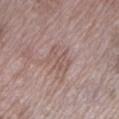The lesion was photographed on a routine skin check and not biopsied; there is no pathology result. Located on the leg. Cropped from a whole-body photographic skin survey; the tile spans about 15 mm. The patient is a female aged 68–72. The total-body-photography lesion software estimated an outline eccentricity of about 0.7 (0 = round, 1 = elongated) and a shape-asymmetry score of about 0.4 (0 = symmetric). The analysis additionally found a mean CIELAB color near L≈54 a*≈17 b*≈22, roughly 6 lightness units darker than nearby skin, and a normalized border contrast of about 5. The software also gave a classifier nevus-likeness of about 0/100 and a detector confidence of about 90 out of 100 that the crop contains a lesion. About 3.5 mm across.